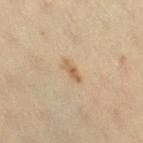No biopsy was performed on this lesion — it was imaged during a full skin examination and was not determined to be concerning.
On the right thigh.
A female subject aged 38–42.
A close-up tile cropped from a whole-body skin photograph, about 15 mm across.